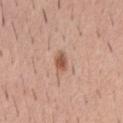Notes:
• follow-up — no biopsy performed (imaged during a skin exam)
• image source — total-body-photography crop, ~15 mm field of view
• lesion size — ~2.5 mm (longest diameter)
• patient — male, about 40 years old
• TBP lesion metrics — a lesion area of about 4 mm² and a shape eccentricity near 0.75
• location — the chest